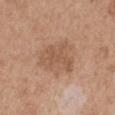| key | value |
|---|---|
| workup | no biopsy performed (imaged during a skin exam) |
| acquisition | 15 mm crop, total-body photography |
| patient | male, aged around 65 |
| location | the arm |
| lighting | white-light |
| lesion diameter | ~5 mm (longest diameter) |